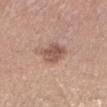Recorded during total-body skin imaging; not selected for excision or biopsy. A female patient, approximately 30 years of age. The lesion's longest dimension is about 3.5 mm. The lesion is located on the leg. The tile uses white-light illumination. A roughly 15 mm field-of-view crop from a total-body skin photograph. Automated image analysis of the tile measured a lesion color around L≈54 a*≈20 b*≈26 in CIELAB and a normalized border contrast of about 7.5. It also reported border irregularity of about 2 on a 0–10 scale, internal color variation of about 3 on a 0–10 scale, and a peripheral color-asymmetry measure near 1. The software also gave a classifier nevus-likeness of about 30/100 and a lesion-detection confidence of about 100/100.